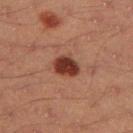Clinical impression: This lesion was catalogued during total-body skin photography and was not selected for biopsy. Background: This is a cross-polarized tile. The subject is a male aged around 45. A roughly 15 mm field-of-view crop from a total-body skin photograph. The recorded lesion diameter is about 3 mm. The lesion is located on the left thigh.